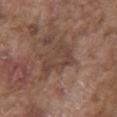Part of a total-body skin-imaging series; this lesion was reviewed on a skin check and was not flagged for biopsy.
The subject is a male approximately 75 years of age.
A 15 mm close-up extracted from a 3D total-body photography capture.
The recorded lesion diameter is about 5 mm.
Captured under white-light illumination.
The lesion is on the abdomen.
An algorithmic analysis of the crop reported a border-irregularity index near 5/10, internal color variation of about 2.5 on a 0–10 scale, and radial color variation of about 0.5. It also reported an automated nevus-likeness rating near 0 out of 100 and a lesion-detection confidence of about 75/100.